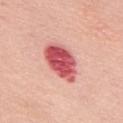Q: Was a biopsy performed?
A: catalogued during a skin exam; not biopsied
Q: Patient demographics?
A: female, in their mid- to late 60s
Q: Lesion size?
A: ~6 mm (longest diameter)
Q: Automated lesion metrics?
A: a shape-asymmetry score of about 0.15 (0 = symmetric); a mean CIELAB color near L≈57 a*≈38 b*≈25 and a lesion–skin lightness drop of about 19; a border-irregularity rating of about 2/10, a color-variation rating of about 6/10, and a peripheral color-asymmetry measure near 2; a classifier nevus-likeness of about 0/100 and lesion-presence confidence of about 100/100
Q: What lighting was used for the tile?
A: white-light illumination
Q: What is the imaging modality?
A: total-body-photography crop, ~15 mm field of view
Q: Lesion location?
A: the back